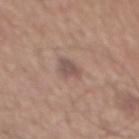Assessment: This lesion was catalogued during total-body skin photography and was not selected for biopsy. Acquisition and patient details: The patient is a male approximately 65 years of age. Located on the mid back. A close-up tile cropped from a whole-body skin photograph, about 15 mm across. This is a white-light tile.